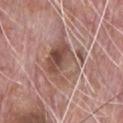Image and clinical context:
A 15 mm close-up extracted from a 3D total-body photography capture. Imaged with white-light lighting. The lesion is located on the chest. Approximately 6.5 mm at its widest. The patient is a male aged 68 to 72.